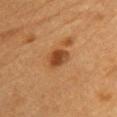The lesion was photographed on a routine skin check and not biopsied; there is no pathology result. The subject is a male aged approximately 85 or older. The tile uses cross-polarized illumination. The lesion-visualizer software estimated an outline eccentricity of about 0.65 (0 = round, 1 = elongated). It also reported a border-irregularity index near 2/10, a within-lesion color-variation index near 4.5/10, and a peripheral color-asymmetry measure near 1.5. And it measured a nevus-likeness score of about 80/100 and lesion-presence confidence of about 100/100. Approximately 2.5 mm at its widest. A 15 mm close-up extracted from a 3D total-body photography capture. The lesion is on the chest.